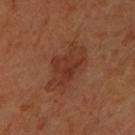| key | value |
|---|---|
| workup | catalogued during a skin exam; not biopsied |
| imaging modality | total-body-photography crop, ~15 mm field of view |
| subject | female |
| site | the left forearm |